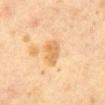<record>
  <biopsy_status>not biopsied; imaged during a skin examination</biopsy_status>
  <patient>
    <sex>male</sex>
    <age_approx>60</age_approx>
  </patient>
  <site>abdomen</site>
  <lesion_size>
    <long_diameter_mm_approx>3.5</long_diameter_mm_approx>
  </lesion_size>
  <automated_metrics>
    <cielab_L>57</cielab_L>
    <cielab_a>18</cielab_a>
    <cielab_b>37</cielab_b>
    <vs_skin_contrast_norm>6.5</vs_skin_contrast_norm>
    <border_irregularity_0_10>2.0</border_irregularity_0_10>
    <color_variation_0_10>2.5</color_variation_0_10>
    <peripheral_color_asymmetry>1.0</peripheral_color_asymmetry>
    <nevus_likeness_0_100>0</nevus_likeness_0_100>
    <lesion_detection_confidence_0_100>100</lesion_detection_confidence_0_100>
  </automated_metrics>
  <image>
    <source>total-body photography crop</source>
    <field_of_view_mm>15</field_of_view_mm>
  </image>
  <lighting>cross-polarized</lighting>
</record>